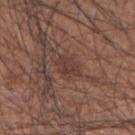Assessment:
Part of a total-body skin-imaging series; this lesion was reviewed on a skin check and was not flagged for biopsy.
Context:
From the arm. A male subject, aged 33 to 37. A region of skin cropped from a whole-body photographic capture, roughly 15 mm wide.